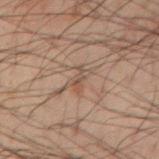Q: Is there a histopathology result?
A: no biopsy performed (imaged during a skin exam)
Q: What is the anatomic site?
A: the left forearm
Q: What is the lesion's diameter?
A: ≈3 mm
Q: Patient demographics?
A: male, roughly 40 years of age
Q: What lighting was used for the tile?
A: cross-polarized illumination
Q: What is the imaging modality?
A: ~15 mm tile from a whole-body skin photo